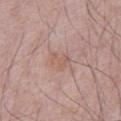Assessment:
Part of a total-body skin-imaging series; this lesion was reviewed on a skin check and was not flagged for biopsy.
Clinical summary:
A 15 mm crop from a total-body photograph taken for skin-cancer surveillance. Measured at roughly 2.5 mm in maximum diameter. The total-body-photography lesion software estimated an area of roughly 4.5 mm² and an eccentricity of roughly 0.65. The software also gave an automated nevus-likeness rating near 0 out of 100 and lesion-presence confidence of about 100/100. The subject is a male aged 63–67. From the abdomen. This is a white-light tile.